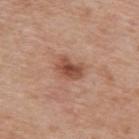<record>
<biopsy_status>not biopsied; imaged during a skin examination</biopsy_status>
<patient>
  <sex>female</sex>
  <age_approx>40</age_approx>
</patient>
<automated_metrics>
  <eccentricity>0.7</eccentricity>
  <shape_asymmetry>0.3</shape_asymmetry>
  <cielab_L>50</cielab_L>
  <cielab_a>23</cielab_a>
  <cielab_b>30</cielab_b>
  <vs_skin_darker_L>12.0</vs_skin_darker_L>
  <vs_skin_contrast_norm>8.0</vs_skin_contrast_norm>
  <color_variation_0_10>4.0</color_variation_0_10>
  <peripheral_color_asymmetry>1.0</peripheral_color_asymmetry>
  <nevus_likeness_0_100>80</nevus_likeness_0_100>
  <lesion_detection_confidence_0_100>100</lesion_detection_confidence_0_100>
</automated_metrics>
<site>back</site>
<image>
  <source>total-body photography crop</source>
  <field_of_view_mm>15</field_of_view_mm>
</image>
<lighting>white-light</lighting>
</record>